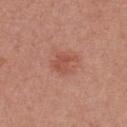The lesion was tiled from a total-body skin photograph and was not biopsied. Captured under white-light illumination. A female patient in their mid-20s. Measured at roughly 3 mm in maximum diameter. This image is a 15 mm lesion crop taken from a total-body photograph. Located on the front of the torso.